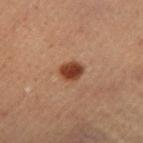Recorded during total-body skin imaging; not selected for excision or biopsy.
Imaged with cross-polarized lighting.
A 15 mm close-up extracted from a 3D total-body photography capture.
The lesion is located on the leg.
Longest diameter approximately 3 mm.
A male patient, roughly 50 years of age.